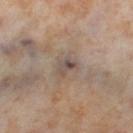{"biopsy_status": "not biopsied; imaged during a skin examination", "image": {"source": "total-body photography crop", "field_of_view_mm": 15}, "site": "right thigh", "patient": {"sex": "female", "age_approx": 55}, "lesion_size": {"long_diameter_mm_approx": 2.5}, "lighting": "cross-polarized"}